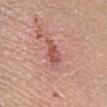| feature | finding |
|---|---|
| workup | no biopsy performed (imaged during a skin exam) |
| size | ~4 mm (longest diameter) |
| imaging modality | ~15 mm tile from a whole-body skin photo |
| image-analysis metrics | an automated nevus-likeness rating near 20 out of 100 and a lesion-detection confidence of about 100/100 |
| anatomic site | the right lower leg |
| tile lighting | white-light |
| patient | female, aged 48 to 52 |